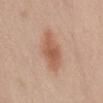Part of a total-body skin-imaging series; this lesion was reviewed on a skin check and was not flagged for biopsy.
A female patient, aged around 50.
The lesion's longest dimension is about 6 mm.
On the front of the torso.
A 15 mm crop from a total-body photograph taken for skin-cancer surveillance.